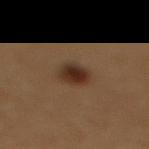workup: imaged on a skin check; not biopsied
TBP lesion metrics: an eccentricity of roughly 0.75 and a symmetry-axis asymmetry near 0.2; a lesion color around L≈26 a*≈15 b*≈23 in CIELAB, about 10 CIELAB-L* units darker than the surrounding skin, and a lesion-to-skin contrast of about 10 (normalized; higher = more distinct); a border-irregularity index near 2/10, internal color variation of about 4 on a 0–10 scale, and a peripheral color-asymmetry measure near 1
illumination: cross-polarized illumination
patient: female, approximately 50 years of age
lesion diameter: about 3.5 mm
body site: the upper back
image source: 15 mm crop, total-body photography